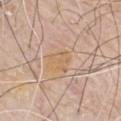Notes:
- biopsy status: no biopsy performed (imaged during a skin exam)
- diameter: about 3 mm
- acquisition: ~15 mm crop, total-body skin-cancer survey
- tile lighting: white-light illumination
- anatomic site: the front of the torso
- patient: male, aged 78 to 82